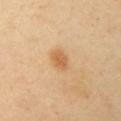notes: no biopsy performed (imaged during a skin exam) | diameter: ~3 mm (longest diameter) | subject: female, aged 38–42 | imaging modality: ~15 mm tile from a whole-body skin photo | anatomic site: the left upper arm.